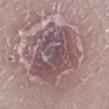patient = female, aged 58–62; tile lighting = white-light illumination; body site = the left lower leg; image source = total-body-photography crop, ~15 mm field of view; lesion size = ~8.5 mm (longest diameter).